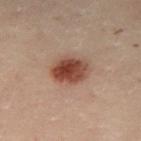Clinical impression: No biopsy was performed on this lesion — it was imaged during a full skin examination and was not determined to be concerning. Background: The subject is a female aged 28 to 32. From the left leg. A lesion tile, about 15 mm wide, cut from a 3D total-body photograph.